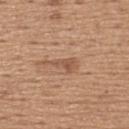Imaged during a routine full-body skin examination; the lesion was not biopsied and no histopathology is available. The lesion is located on the upper back. Measured at roughly 4 mm in maximum diameter. Cropped from a total-body skin-imaging series; the visible field is about 15 mm. An algorithmic analysis of the crop reported a lesion area of about 4.5 mm² and an eccentricity of roughly 0.95. The analysis additionally found a border-irregularity index near 6.5/10 and peripheral color asymmetry of about 0.5. The subject is a male about 65 years old.